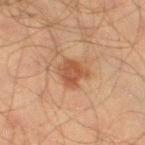biopsy status: no biopsy performed (imaged during a skin exam) | illumination: cross-polarized | body site: the left thigh | acquisition: total-body-photography crop, ~15 mm field of view | TBP lesion metrics: roughly 9 lightness units darker than nearby skin and a lesion-to-skin contrast of about 7 (normalized; higher = more distinct); an automated nevus-likeness rating near 75 out of 100 and a detector confidence of about 100 out of 100 that the crop contains a lesion | size: ≈3 mm | subject: male, aged 43 to 47.